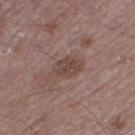No biopsy was performed on this lesion — it was imaged during a full skin examination and was not determined to be concerning. The tile uses white-light illumination. A male subject, aged 48–52. A close-up tile cropped from a whole-body skin photograph, about 15 mm across. The lesion is on the left thigh. Automated image analysis of the tile measured a shape-asymmetry score of about 0.15 (0 = symmetric). The software also gave an average lesion color of about L≈44 a*≈18 b*≈21 (CIELAB).